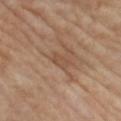Q: How large is the lesion?
A: ≈3 mm
Q: What kind of image is this?
A: ~15 mm tile from a whole-body skin photo
Q: Where on the body is the lesion?
A: the left lower leg
Q: How was the tile lit?
A: cross-polarized
Q: What are the patient's age and sex?
A: female, about 70 years old